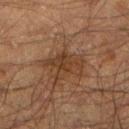Assessment: The lesion was photographed on a routine skin check and not biopsied; there is no pathology result. Clinical summary: Approximately 4.5 mm at its widest. The lesion-visualizer software estimated a lesion area of about 9.5 mm², an outline eccentricity of about 0.75 (0 = round, 1 = elongated), and two-axis asymmetry of about 0.4. And it measured an average lesion color of about L≈29 a*≈15 b*≈25 (CIELAB) and a lesion–skin lightness drop of about 6. The analysis additionally found a border-irregularity rating of about 6/10, a color-variation rating of about 2.5/10, and peripheral color asymmetry of about 1. A male patient approximately 50 years of age. A 15 mm close-up tile from a total-body photography series done for melanoma screening. The lesion is on the leg. Imaged with cross-polarized lighting.